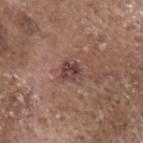Clinical impression:
Captured during whole-body skin photography for melanoma surveillance; the lesion was not biopsied.
Background:
The lesion is located on the head or neck. The total-body-photography lesion software estimated an area of roughly 5 mm², a shape eccentricity near 0.3, and a symmetry-axis asymmetry near 0.3. It also reported a border-irregularity index near 3/10, a color-variation rating of about 5/10, and peripheral color asymmetry of about 2. The software also gave a nevus-likeness score of about 50/100 and a detector confidence of about 100 out of 100 that the crop contains a lesion. Longest diameter approximately 2.5 mm. Imaged with white-light lighting. A male patient in their 80s. A 15 mm close-up tile from a total-body photography series done for melanoma screening.